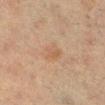Clinical impression:
The lesion was photographed on a routine skin check and not biopsied; there is no pathology result.
Context:
A female subject, in their mid- to late 50s. This is a cross-polarized tile. From the leg. About 3 mm across. Cropped from a whole-body photographic skin survey; the tile spans about 15 mm.